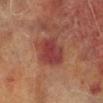Assessment:
The lesion was tiled from a total-body skin photograph and was not biopsied.
Context:
A male patient, aged 68–72. Longest diameter approximately 4 mm. A close-up tile cropped from a whole-body skin photograph, about 15 mm across. On the leg. Captured under cross-polarized illumination. Automated tile analysis of the lesion measured a lesion area of about 11 mm², an eccentricity of roughly 0.55, and a symmetry-axis asymmetry near 0.2. The software also gave a mean CIELAB color near L≈32 a*≈25 b*≈21, roughly 8 lightness units darker than nearby skin, and a normalized lesion–skin contrast near 8. And it measured a border-irregularity index near 2/10, a color-variation rating of about 3/10, and peripheral color asymmetry of about 1.